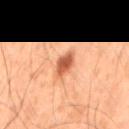Captured during whole-body skin photography for melanoma surveillance; the lesion was not biopsied.
A male patient, in their 50s.
Automated tile analysis of the lesion measured an area of roughly 6 mm², a shape eccentricity near 0.8, and two-axis asymmetry of about 0.2. The software also gave an average lesion color of about L≈46 a*≈23 b*≈31 (CIELAB), a lesion–skin lightness drop of about 12, and a normalized lesion–skin contrast near 9.
This image is a 15 mm lesion crop taken from a total-body photograph.
Captured under cross-polarized illumination.
On the mid back.
The lesion's longest dimension is about 3.5 mm.